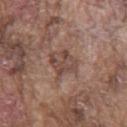Findings:
- notes — imaged on a skin check; not biopsied
- location — the chest
- patient — male, in their mid-70s
- lesion size — about 4 mm
- image source — total-body-photography crop, ~15 mm field of view
- automated metrics — a footprint of about 8.5 mm² and two-axis asymmetry of about 0.25